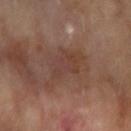<lesion>
  <biopsy_status>not biopsied; imaged during a skin examination</biopsy_status>
  <site>right forearm</site>
  <lighting>cross-polarized</lighting>
  <image>
    <source>total-body photography crop</source>
    <field_of_view_mm>15</field_of_view_mm>
  </image>
  <patient>
    <sex>female</sex>
    <age_approx>60</age_approx>
  </patient>
</lesion>